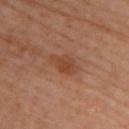workup: total-body-photography surveillance lesion; no biopsy
size: about 3.5 mm
subject: female, aged around 60
automated lesion analysis: a mean CIELAB color near L≈45 a*≈24 b*≈34 and a lesion-to-skin contrast of about 7 (normalized; higher = more distinct); lesion-presence confidence of about 100/100
location: the upper back
imaging modality: 15 mm crop, total-body photography
tile lighting: cross-polarized illumination